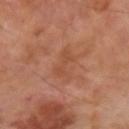Captured during whole-body skin photography for melanoma surveillance; the lesion was not biopsied.